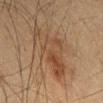Assessment: Imaged during a routine full-body skin examination; the lesion was not biopsied and no histopathology is available. Background: Cropped from a whole-body photographic skin survey; the tile spans about 15 mm. Automated image analysis of the tile measured a lesion–skin lightness drop of about 7 and a normalized border contrast of about 6. The recorded lesion diameter is about 7.5 mm. The subject is a male about 65 years old. Captured under cross-polarized illumination. From the back.